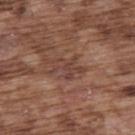{"biopsy_status": "not biopsied; imaged during a skin examination", "image": {"source": "total-body photography crop", "field_of_view_mm": 15}, "lesion_size": {"long_diameter_mm_approx": 3.5}, "site": "upper back", "patient": {"sex": "male", "age_approx": 75}}